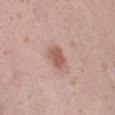This lesion was catalogued during total-body skin photography and was not selected for biopsy. A region of skin cropped from a whole-body photographic capture, roughly 15 mm wide. On the abdomen. About 3 mm across. A female patient, approximately 40 years of age.